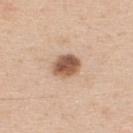{
  "biopsy_status": "not biopsied; imaged during a skin examination",
  "site": "upper back",
  "patient": {
    "sex": "male",
    "age_approx": 35
  },
  "image": {
    "source": "total-body photography crop",
    "field_of_view_mm": 15
  }
}